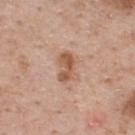  biopsy_status: not biopsied; imaged during a skin examination
  automated_metrics:
    area_mm2_approx: 6.5
    eccentricity: 0.8
    shape_asymmetry: 0.25
    cielab_L: 57
    cielab_a: 20
    cielab_b: 32
    vs_skin_darker_L: 11.0
    vs_skin_contrast_norm: 7.5
    color_variation_0_10: 4.0
    peripheral_color_asymmetry: 1.5
    nevus_likeness_0_100: 10
  patient:
    sex: male
    age_approx: 60
  site: upper back
  lesion_size:
    long_diameter_mm_approx: 3.5
  image:
    source: total-body photography crop
    field_of_view_mm: 15
  lighting: white-light